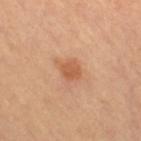The lesion was photographed on a routine skin check and not biopsied; there is no pathology result. A female patient aged around 60. Longest diameter approximately 2.5 mm. Captured under cross-polarized illumination. A roughly 15 mm field-of-view crop from a total-body skin photograph. The total-body-photography lesion software estimated a lesion color around L≈58 a*≈26 b*≈37 in CIELAB, a lesion–skin lightness drop of about 10, and a normalized lesion–skin contrast near 6.5. It also reported a classifier nevus-likeness of about 70/100 and a lesion-detection confidence of about 100/100. Located on the right thigh.